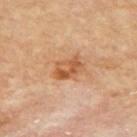Case summary:
• follow-up — no biopsy performed (imaged during a skin exam)
• acquisition — 15 mm crop, total-body photography
• site — the upper back
• patient — male, aged approximately 85 or older
• lighting — cross-polarized illumination